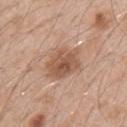lesion diameter: about 4 mm; image: total-body-photography crop, ~15 mm field of view; lighting: white-light illumination; patient: male, approximately 50 years of age; location: the left upper arm.